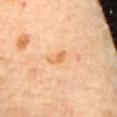{"biopsy_status": "not biopsied; imaged during a skin examination", "patient": {"sex": "female", "age_approx": 65}, "lighting": "cross-polarized", "site": "mid back", "automated_metrics": {"border_irregularity_0_10": 4.0, "color_variation_0_10": 0.0, "nevus_likeness_0_100": 0}, "image": {"source": "total-body photography crop", "field_of_view_mm": 15}, "lesion_size": {"long_diameter_mm_approx": 3.0}}